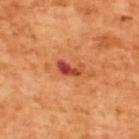notes: imaged on a skin check; not biopsied | lighting: cross-polarized illumination | acquisition: 15 mm crop, total-body photography | patient: male, aged around 65 | body site: the upper back | diameter: ~3 mm (longest diameter) | automated lesion analysis: an average lesion color of about L≈46 a*≈35 b*≈36 (CIELAB), about 14 CIELAB-L* units darker than the surrounding skin, and a normalized border contrast of about 10; a nevus-likeness score of about 0/100.